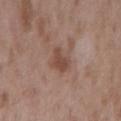Captured during whole-body skin photography for melanoma surveillance; the lesion was not biopsied. Measured at roughly 3 mm in maximum diameter. This image is a 15 mm lesion crop taken from a total-body photograph. Imaged with white-light lighting. Located on the arm. The subject is a male approximately 50 years of age. The lesion-visualizer software estimated an area of roughly 5 mm², a shape eccentricity near 0.8, and a symmetry-axis asymmetry near 0.4. It also reported an average lesion color of about L≈46 a*≈19 b*≈25 (CIELAB), roughly 9 lightness units darker than nearby skin, and a normalized lesion–skin contrast near 7. And it measured a border-irregularity rating of about 4/10, a color-variation rating of about 1.5/10, and peripheral color asymmetry of about 0.5. It also reported an automated nevus-likeness rating near 0 out of 100 and lesion-presence confidence of about 100/100.